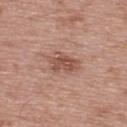lighting: white-light illumination | image: ~15 mm crop, total-body skin-cancer survey | automated metrics: a shape eccentricity near 0.75 and a symmetry-axis asymmetry near 0.2; a mean CIELAB color near L≈52 a*≈22 b*≈27; a border-irregularity index near 3/10, a within-lesion color-variation index near 3/10, and peripheral color asymmetry of about 1; a nevus-likeness score of about 20/100 | subject: male, in their mid-60s | location: the upper back | size: about 4 mm.